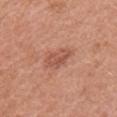Imaged during a routine full-body skin examination; the lesion was not biopsied and no histopathology is available. A lesion tile, about 15 mm wide, cut from a 3D total-body photograph. Automated tile analysis of the lesion measured a footprint of about 6 mm² and a shape-asymmetry score of about 0.3 (0 = symmetric). It also reported internal color variation of about 3.5 on a 0–10 scale and a peripheral color-asymmetry measure near 1.5. And it measured an automated nevus-likeness rating near 20 out of 100 and a lesion-detection confidence of about 100/100. The subject is a male in their 70s. From the left upper arm. About 3 mm across.